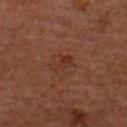Impression:
Imaged during a routine full-body skin examination; the lesion was not biopsied and no histopathology is available.
Context:
Approximately 3 mm at its widest. Captured under cross-polarized illumination. The total-body-photography lesion software estimated an area of roughly 3.5 mm², a shape eccentricity near 0.85, and a symmetry-axis asymmetry near 0.35. A 15 mm crop from a total-body photograph taken for skin-cancer surveillance. On the chest. The subject is a male in their 60s.